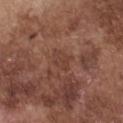Part of a total-body skin-imaging series; this lesion was reviewed on a skin check and was not flagged for biopsy. About 2.5 mm across. From the front of the torso. Imaged with white-light lighting. The patient is a male about 75 years old. This image is a 15 mm lesion crop taken from a total-body photograph.